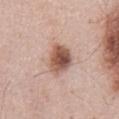Impression: This lesion was catalogued during total-body skin photography and was not selected for biopsy. Image and clinical context: The lesion is on the abdomen. Imaged with white-light lighting. A 15 mm close-up tile from a total-body photography series done for melanoma screening. A male subject aged 53 to 57.